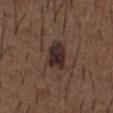Clinical impression: The lesion was photographed on a routine skin check and not biopsied; there is no pathology result. Clinical summary: A male subject about 50 years old. An algorithmic analysis of the crop reported an eccentricity of roughly 0.7 and a shape-asymmetry score of about 0.2 (0 = symmetric). And it measured an average lesion color of about L≈28 a*≈15 b*≈18 (CIELAB). It also reported a border-irregularity rating of about 2/10 and a peripheral color-asymmetry measure near 2. A roughly 15 mm field-of-view crop from a total-body skin photograph. From the chest.